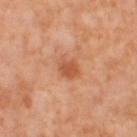patient: female, approximately 55 years of age; image: ~15 mm crop, total-body skin-cancer survey; body site: the left thigh; lighting: cross-polarized.